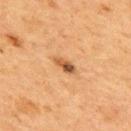– workup: no biopsy performed (imaged during a skin exam)
– illumination: cross-polarized illumination
– lesion size: ≈3 mm
– patient: male, in their mid- to late 60s
– body site: the upper back
– imaging modality: total-body-photography crop, ~15 mm field of view